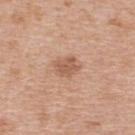biopsy status: no biopsy performed (imaged during a skin exam)
tile lighting: white-light
image: 15 mm crop, total-body photography
lesion size: about 2.5 mm
automated metrics: a footprint of about 5 mm² and a shape-asymmetry score of about 0.25 (0 = symmetric); an average lesion color of about L≈57 a*≈21 b*≈31 (CIELAB), roughly 10 lightness units darker than nearby skin, and a lesion-to-skin contrast of about 7 (normalized; higher = more distinct); a border-irregularity index near 2.5/10, a color-variation rating of about 1.5/10, and a peripheral color-asymmetry measure near 0.5; an automated nevus-likeness rating near 10 out of 100 and a detector confidence of about 100 out of 100 that the crop contains a lesion
patient: female, approximately 40 years of age
anatomic site: the upper back